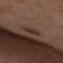Assessment: Part of a total-body skin-imaging series; this lesion was reviewed on a skin check and was not flagged for biopsy. Clinical summary: The lesion is on the left upper arm. Measured at roughly 3 mm in maximum diameter. A 15 mm close-up tile from a total-body photography series done for melanoma screening. The lesion-visualizer software estimated a shape eccentricity near 0.85 and a shape-asymmetry score of about 0.25 (0 = symmetric). And it measured a lesion–skin lightness drop of about 7. The software also gave border irregularity of about 3.5 on a 0–10 scale, a color-variation rating of about 1/10, and radial color variation of about 0.5. And it measured an automated nevus-likeness rating near 40 out of 100 and a detector confidence of about 100 out of 100 that the crop contains a lesion. Captured under white-light illumination. A female subject approximately 35 years of age.